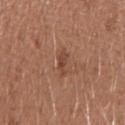Image and clinical context: This is a white-light tile. The recorded lesion diameter is about 2.5 mm. From the left upper arm. A 15 mm crop from a total-body photograph taken for skin-cancer surveillance. A female subject aged 33 to 37.